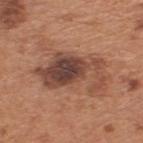follow-up: catalogued during a skin exam; not biopsied | location: the back | subject: male, about 65 years old | image: ~15 mm crop, total-body skin-cancer survey.